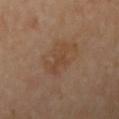Q: Is there a histopathology result?
A: imaged on a skin check; not biopsied
Q: What kind of image is this?
A: ~15 mm tile from a whole-body skin photo
Q: Automated lesion metrics?
A: a lesion area of about 5 mm², an outline eccentricity of about 0.85 (0 = round, 1 = elongated), and two-axis asymmetry of about 0.45; about 5 CIELAB-L* units darker than the surrounding skin and a normalized lesion–skin contrast near 5; a within-lesion color-variation index near 1/10; a nevus-likeness score of about 0/100 and lesion-presence confidence of about 100/100
Q: How was the tile lit?
A: cross-polarized illumination
Q: Patient demographics?
A: female, aged approximately 65
Q: Lesion size?
A: about 3.5 mm
Q: Where on the body is the lesion?
A: the right upper arm